A 15 mm crop from a total-body photograph taken for skin-cancer surveillance; a female patient, in their mid-60s; from the left thigh:
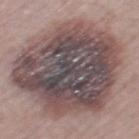| key | value |
|---|---|
| histopathology | an invasive melanoma, superficial spreading type (Breslow thickness 0.6 mm, mitotic rate <1/mm²) |